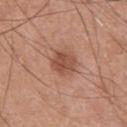No biopsy was performed on this lesion — it was imaged during a full skin examination and was not determined to be concerning. The tile uses white-light illumination. An algorithmic analysis of the crop reported a mean CIELAB color near L≈50 a*≈24 b*≈30, roughly 10 lightness units darker than nearby skin, and a normalized lesion–skin contrast near 7.5. The analysis additionally found an automated nevus-likeness rating near 55 out of 100 and a lesion-detection confidence of about 100/100. Measured at roughly 3 mm in maximum diameter. The lesion is located on the chest. A close-up tile cropped from a whole-body skin photograph, about 15 mm across. The subject is a male aged 53–57.